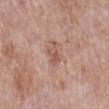Assessment: This lesion was catalogued during total-body skin photography and was not selected for biopsy. Image and clinical context: A 15 mm crop from a total-body photograph taken for skin-cancer surveillance. The patient is a female approximately 70 years of age. The lesion is on the right lower leg.